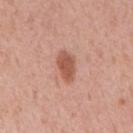Clinical impression: Recorded during total-body skin imaging; not selected for excision or biopsy. Background: Located on the mid back. A 15 mm close-up extracted from a 3D total-body photography capture. A male patient approximately 55 years of age.